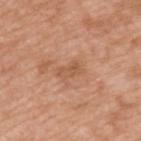| field | value |
|---|---|
| biopsy status | total-body-photography surveillance lesion; no biopsy |
| lesion diameter | about 3 mm |
| body site | the upper back |
| patient | male, aged approximately 50 |
| image-analysis metrics | a border-irregularity index near 3/10 and a color-variation rating of about 3/10 |
| illumination | white-light illumination |
| image source | 15 mm crop, total-body photography |